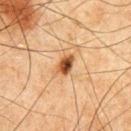Part of a total-body skin-imaging series; this lesion was reviewed on a skin check and was not flagged for biopsy.
Longest diameter approximately 3 mm.
A close-up tile cropped from a whole-body skin photograph, about 15 mm across.
The tile uses cross-polarized illumination.
Automated image analysis of the tile measured a lesion color around L≈45 a*≈22 b*≈35 in CIELAB and about 16 CIELAB-L* units darker than the surrounding skin. And it measured an automated nevus-likeness rating near 100 out of 100.
A male subject, approximately 65 years of age.
From the abdomen.